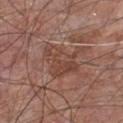biopsy status — catalogued during a skin exam; not biopsied | lesion size — ~4.5 mm (longest diameter) | image-analysis metrics — an area of roughly 10 mm², a shape eccentricity near 0.75, and a shape-asymmetry score of about 0.45 (0 = symmetric); a lesion–skin lightness drop of about 7 and a lesion-to-skin contrast of about 5.5 (normalized; higher = more distinct); a border-irregularity rating of about 5.5/10, internal color variation of about 4 on a 0–10 scale, and a peripheral color-asymmetry measure near 1 | patient — male, aged 63–67 | image — ~15 mm crop, total-body skin-cancer survey | tile lighting — white-light illumination | location — the chest.